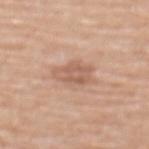No biopsy was performed on this lesion — it was imaged during a full skin examination and was not determined to be concerning. This is a white-light tile. A roughly 15 mm field-of-view crop from a total-body skin photograph. The lesion is located on the upper back. About 4 mm across. The patient is a female roughly 70 years of age.